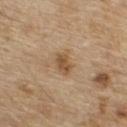Clinical impression: Captured during whole-body skin photography for melanoma surveillance; the lesion was not biopsied. Context: The lesion is located on the front of the torso. Measured at roughly 3 mm in maximum diameter. A 15 mm close-up tile from a total-body photography series done for melanoma screening. A male subject, aged around 70.